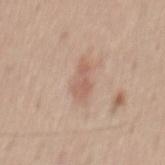follow-up: imaged on a skin check; not biopsied.